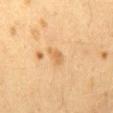This lesion was catalogued during total-body skin photography and was not selected for biopsy.